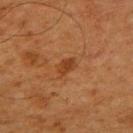{
  "biopsy_status": "not biopsied; imaged during a skin examination",
  "lesion_size": {
    "long_diameter_mm_approx": 2.5
  },
  "lighting": "cross-polarized",
  "patient": {
    "sex": "male",
    "age_approx": 65
  },
  "site": "upper back",
  "image": {
    "source": "total-body photography crop",
    "field_of_view_mm": 15
  }
}